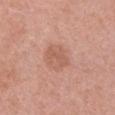Impression:
Recorded during total-body skin imaging; not selected for excision or biopsy.
Acquisition and patient details:
A region of skin cropped from a whole-body photographic capture, roughly 15 mm wide. This is a white-light tile. The patient is a female approximately 45 years of age. The lesion is located on the left upper arm.